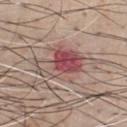Assessment: The lesion was tiled from a total-body skin photograph and was not biopsied. Background: The lesion's longest dimension is about 8 mm. A male subject about 40 years old. A region of skin cropped from a whole-body photographic capture, roughly 15 mm wide. The lesion is on the chest.